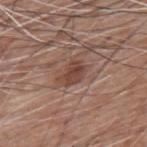| key | value |
|---|---|
| biopsy status | imaged on a skin check; not biopsied |
| automated lesion analysis | a footprint of about 6 mm², an outline eccentricity of about 0.75 (0 = round, 1 = elongated), and a symmetry-axis asymmetry near 0.25; an automated nevus-likeness rating near 50 out of 100 and a detector confidence of about 100 out of 100 that the crop contains a lesion |
| illumination | white-light |
| acquisition | total-body-photography crop, ~15 mm field of view |
| patient | male, approximately 60 years of age |
| site | the upper back |
| lesion diameter | ≈3.5 mm |